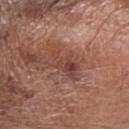lighting — white-light | subject — male, about 70 years old | automated metrics — a symmetry-axis asymmetry near 0.3; a mean CIELAB color near L≈43 a*≈22 b*≈25 and a lesion–skin lightness drop of about 8; a border-irregularity index near 4.5/10 and a color-variation rating of about 7.5/10 | body site — the left forearm | diameter — about 4.5 mm | image source — total-body-photography crop, ~15 mm field of view.